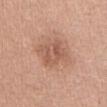The lesion was tiled from a total-body skin photograph and was not biopsied. A female patient, in their mid-30s. Imaged with white-light lighting. This image is a 15 mm lesion crop taken from a total-body photograph. The lesion is located on the front of the torso. The lesion's longest dimension is about 4 mm.